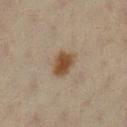{"biopsy_status": "not biopsied; imaged during a skin examination", "site": "left lower leg", "lesion_size": {"long_diameter_mm_approx": 3.5}, "lighting": "cross-polarized", "image": {"source": "total-body photography crop", "field_of_view_mm": 15}, "patient": {"sex": "female", "age_approx": 45}}